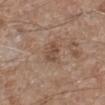The lesion was tiled from a total-body skin photograph and was not biopsied. A roughly 15 mm field-of-view crop from a total-body skin photograph. About 2.5 mm across. Located on the left lower leg. Captured under white-light illumination. A male patient aged 63 to 67.